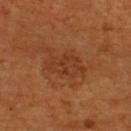Q: Was this lesion biopsied?
A: no biopsy performed (imaged during a skin exam)
Q: What is the imaging modality?
A: total-body-photography crop, ~15 mm field of view
Q: What is the lesion's diameter?
A: about 5 mm
Q: What did automated image analysis measure?
A: an area of roughly 10 mm², an outline eccentricity of about 0.8 (0 = round, 1 = elongated), and a shape-asymmetry score of about 0.25 (0 = symmetric); a lesion color around L≈34 a*≈23 b*≈32 in CIELAB, a lesion–skin lightness drop of about 6, and a normalized lesion–skin contrast near 5.5; border irregularity of about 3 on a 0–10 scale, internal color variation of about 2.5 on a 0–10 scale, and radial color variation of about 1
Q: What are the patient's age and sex?
A: male, aged approximately 55
Q: What lighting was used for the tile?
A: cross-polarized illumination
Q: Where on the body is the lesion?
A: the upper back